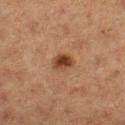Clinical impression: Recorded during total-body skin imaging; not selected for excision or biopsy. Image and clinical context: A female subject about 40 years old. This is a cross-polarized tile. On the right lower leg. This image is a 15 mm lesion crop taken from a total-body photograph. Approximately 2.5 mm at its widest.